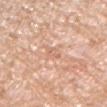| field | value |
|---|---|
| follow-up | imaged on a skin check; not biopsied |
| lesion diameter | ≈3 mm |
| location | the right upper arm |
| patient | male, aged 58–62 |
| illumination | white-light illumination |
| image source | 15 mm crop, total-body photography |